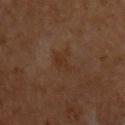notes = catalogued during a skin exam; not biopsied
acquisition = ~15 mm crop, total-body skin-cancer survey
patient = male, about 60 years old
location = the chest